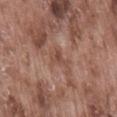Assessment:
The lesion was tiled from a total-body skin photograph and was not biopsied.
Acquisition and patient details:
An algorithmic analysis of the crop reported a lesion area of about 3 mm², a shape eccentricity near 0.8, and a shape-asymmetry score of about 0.55 (0 = symmetric). The analysis additionally found a mean CIELAB color near L≈47 a*≈21 b*≈27, about 7 CIELAB-L* units darker than the surrounding skin, and a lesion-to-skin contrast of about 5.5 (normalized; higher = more distinct). The analysis additionally found border irregularity of about 5 on a 0–10 scale and internal color variation of about 1 on a 0–10 scale. It also reported a nevus-likeness score of about 0/100 and lesion-presence confidence of about 85/100. The tile uses white-light illumination. On the lower back. A 15 mm close-up tile from a total-body photography series done for melanoma screening. The subject is a male roughly 75 years of age.